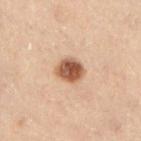Q: Is there a histopathology result?
A: imaged on a skin check; not biopsied
Q: Lesion size?
A: ~3.5 mm (longest diameter)
Q: How was this image acquired?
A: ~15 mm crop, total-body skin-cancer survey
Q: Patient demographics?
A: male, about 55 years old
Q: Where on the body is the lesion?
A: the left lower leg